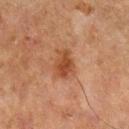follow-up = no biopsy performed (imaged during a skin exam); location = the left lower leg; size = ≈3.5 mm; illumination = cross-polarized illumination; subject = male, aged 63 to 67; image = 15 mm crop, total-body photography.